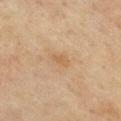Assessment: Part of a total-body skin-imaging series; this lesion was reviewed on a skin check and was not flagged for biopsy.